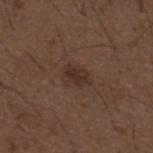Impression: No biopsy was performed on this lesion — it was imaged during a full skin examination and was not determined to be concerning. Context: Cropped from a whole-body photographic skin survey; the tile spans about 15 mm. About 3 mm across. The patient is a male aged 48–52. The lesion is located on the mid back. The lesion-visualizer software estimated a symmetry-axis asymmetry near 0.25. And it measured an average lesion color of about L≈30 a*≈16 b*≈22 (CIELAB), roughly 7 lightness units darker than nearby skin, and a normalized lesion–skin contrast near 7. It also reported a within-lesion color-variation index near 2/10.